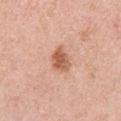No biopsy was performed on this lesion — it was imaged during a full skin examination and was not determined to be concerning. Imaged with white-light lighting. A female patient in their 30s. The total-body-photography lesion software estimated an area of roughly 6 mm² and a shape-asymmetry score of about 0.3 (0 = symmetric). The analysis additionally found an average lesion color of about L≈59 a*≈25 b*≈34 (CIELAB). It also reported radial color variation of about 1.5. The analysis additionally found a nevus-likeness score of about 90/100 and a detector confidence of about 100 out of 100 that the crop contains a lesion. Located on the right upper arm. A roughly 15 mm field-of-view crop from a total-body skin photograph. Measured at roughly 3 mm in maximum diameter.